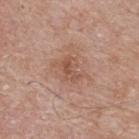Impression:
The lesion was photographed on a routine skin check and not biopsied; there is no pathology result.
Background:
Cropped from a whole-body photographic skin survey; the tile spans about 15 mm. From the upper back. The subject is a male aged 63–67.